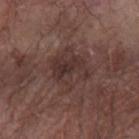<tbp_lesion>
<biopsy_status>not biopsied; imaged during a skin examination</biopsy_status>
<lighting>white-light</lighting>
<lesion_size>
  <long_diameter_mm_approx>4.5</long_diameter_mm_approx>
</lesion_size>
<automated_metrics>
  <cielab_L>33</cielab_L>
  <cielab_a>17</cielab_a>
  <cielab_b>19</cielab_b>
  <vs_skin_darker_L>7.0</vs_skin_darker_L>
</automated_metrics>
<site>left forearm</site>
<image>
  <source>total-body photography crop</source>
  <field_of_view_mm>15</field_of_view_mm>
</image>
<patient>
  <sex>male</sex>
  <age_approx>80</age_approx>
</patient>
</tbp_lesion>